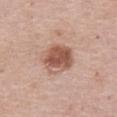The lesion was photographed on a routine skin check and not biopsied; there is no pathology result. The subject is a female roughly 60 years of age. A roughly 15 mm field-of-view crop from a total-body skin photograph. Measured at roughly 4 mm in maximum diameter. Captured under white-light illumination. Located on the abdomen. An algorithmic analysis of the crop reported a lesion area of about 12 mm² and an eccentricity of roughly 0.45. The software also gave about 13 CIELAB-L* units darker than the surrounding skin and a lesion-to-skin contrast of about 9 (normalized; higher = more distinct). And it measured a border-irregularity index near 1.5/10, internal color variation of about 6 on a 0–10 scale, and a peripheral color-asymmetry measure near 2. It also reported a detector confidence of about 100 out of 100 that the crop contains a lesion.